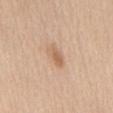biopsy status: total-body-photography surveillance lesion; no biopsy
image source: ~15 mm crop, total-body skin-cancer survey
illumination: white-light illumination
body site: the abdomen
subject: male, in their 60s
lesion size: ≈3 mm